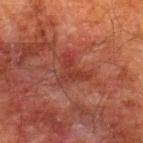On the back.
The subject is a male aged around 60.
Approximately 4 mm at its widest.
Captured under cross-polarized illumination.
A 15 mm close-up tile from a total-body photography series done for melanoma screening.